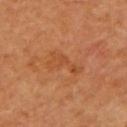Recorded during total-body skin imaging; not selected for excision or biopsy. This is a cross-polarized tile. Located on the left upper arm. Longest diameter approximately 4.5 mm. A 15 mm crop from a total-body photograph taken for skin-cancer surveillance. A female patient, approximately 65 years of age.